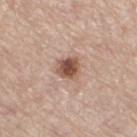{
  "biopsy_status": "not biopsied; imaged during a skin examination",
  "lesion_size": {
    "long_diameter_mm_approx": 3.0
  },
  "patient": {
    "sex": "female",
    "age_approx": 70
  },
  "lighting": "white-light",
  "site": "right thigh",
  "automated_metrics": {
    "border_irregularity_0_10": 3.0,
    "color_variation_0_10": 5.0,
    "peripheral_color_asymmetry": 1.5,
    "nevus_likeness_0_100": 95,
    "lesion_detection_confidence_0_100": 100
  },
  "image": {
    "source": "total-body photography crop",
    "field_of_view_mm": 15
  }
}